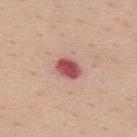Assessment:
Part of a total-body skin-imaging series; this lesion was reviewed on a skin check and was not flagged for biopsy.
Background:
This is a white-light tile. Located on the back. The recorded lesion diameter is about 3 mm. The total-body-photography lesion software estimated a footprint of about 6 mm² and an eccentricity of roughly 0.7. The software also gave a border-irregularity rating of about 1.5/10 and a peripheral color-asymmetry measure near 1.5. A male subject aged 38 to 42. This image is a 15 mm lesion crop taken from a total-body photograph.